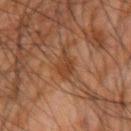Assessment:
The lesion was tiled from a total-body skin photograph and was not biopsied.